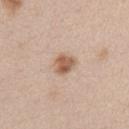Q: Was a biopsy performed?
A: no biopsy performed (imaged during a skin exam)
Q: Patient demographics?
A: female, aged around 40
Q: What is the lesion's diameter?
A: ≈3 mm
Q: What is the imaging modality?
A: ~15 mm tile from a whole-body skin photo
Q: Illumination type?
A: white-light
Q: Where on the body is the lesion?
A: the right upper arm